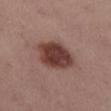Assessment:
The lesion was tiled from a total-body skin photograph and was not biopsied.
Acquisition and patient details:
Captured under white-light illumination. The lesion's longest dimension is about 5 mm. On the left thigh. A lesion tile, about 15 mm wide, cut from a 3D total-body photograph. A female subject, roughly 55 years of age. An algorithmic analysis of the crop reported an area of roughly 15 mm², an eccentricity of roughly 0.6, and two-axis asymmetry of about 0.15. The software also gave a border-irregularity rating of about 1.5/10, a color-variation rating of about 4/10, and peripheral color asymmetry of about 1. The software also gave a classifier nevus-likeness of about 95/100 and a detector confidence of about 100 out of 100 that the crop contains a lesion.